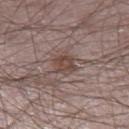Impression: Recorded during total-body skin imaging; not selected for excision or biopsy. Image and clinical context: A male subject, about 65 years old. Captured under white-light illumination. The lesion is on the right thigh. A roughly 15 mm field-of-view crop from a total-body skin photograph. The total-body-photography lesion software estimated an area of roughly 5.5 mm², a shape eccentricity near 0.4, and a symmetry-axis asymmetry near 0.15. And it measured roughly 9 lightness units darker than nearby skin and a normalized lesion–skin contrast near 7.5. About 2.5 mm across.